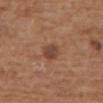Recorded during total-body skin imaging; not selected for excision or biopsy. The recorded lesion diameter is about 3 mm. The lesion is located on the upper back. Automated image analysis of the tile measured a border-irregularity rating of about 1.5/10, a within-lesion color-variation index near 3.5/10, and a peripheral color-asymmetry measure near 1.5. The tile uses white-light illumination. A female patient, aged 73–77. A lesion tile, about 15 mm wide, cut from a 3D total-body photograph.